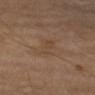Imaged with cross-polarized lighting. The patient is a male roughly 70 years of age. The lesion is on the right forearm. Measured at roughly 3.5 mm in maximum diameter. A 15 mm close-up tile from a total-body photography series done for melanoma screening.